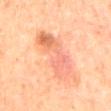  biopsy_status: not biopsied; imaged during a skin examination